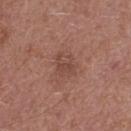Clinical impression: Part of a total-body skin-imaging series; this lesion was reviewed on a skin check and was not flagged for biopsy. Context: Automated image analysis of the tile measured a border-irregularity rating of about 2.5/10, a within-lesion color-variation index near 3/10, and peripheral color asymmetry of about 1. The analysis additionally found a nevus-likeness score of about 0/100. The tile uses white-light illumination. This image is a 15 mm lesion crop taken from a total-body photograph. The recorded lesion diameter is about 3 mm. A female subject, in their 60s. From the back.